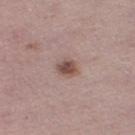The lesion was tiled from a total-body skin photograph and was not biopsied.
An algorithmic analysis of the crop reported a shape eccentricity near 0.55 and a shape-asymmetry score of about 0.25 (0 = symmetric). It also reported an average lesion color of about L≈50 a*≈18 b*≈23 (CIELAB), about 12 CIELAB-L* units darker than the surrounding skin, and a normalized border contrast of about 8.5. It also reported a border-irregularity rating of about 2/10 and radial color variation of about 1.
The lesion is located on the leg.
Captured under white-light illumination.
A 15 mm crop from a total-body photograph taken for skin-cancer surveillance.
A female patient, in their 50s.
About 2.5 mm across.